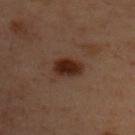{
  "patient": {
    "sex": "female",
    "age_approx": 55
  },
  "site": "upper back",
  "image": {
    "source": "total-body photography crop",
    "field_of_view_mm": 15
  }
}